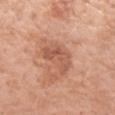lesion size — ≈4.5 mm
location — the left forearm
patient — female, aged 53 to 57
automated lesion analysis — border irregularity of about 9 on a 0–10 scale and a within-lesion color-variation index near 2.5/10
imaging modality — 15 mm crop, total-body photography
lighting — white-light illumination